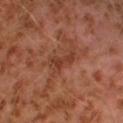Clinical impression: Captured during whole-body skin photography for melanoma surveillance; the lesion was not biopsied. Clinical summary: The tile uses cross-polarized illumination. A male patient approximately 30 years of age. This image is a 15 mm lesion crop taken from a total-body photograph. On the left leg.